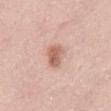This lesion was catalogued during total-body skin photography and was not selected for biopsy. The lesion is located on the left thigh. Automated image analysis of the tile measured a border-irregularity rating of about 2/10 and radial color variation of about 1. A roughly 15 mm field-of-view crop from a total-body skin photograph. The subject is a female about 65 years old. Imaged with white-light lighting. The lesion's longest dimension is about 3.5 mm.